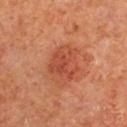The lesion was photographed on a routine skin check and not biopsied; there is no pathology result.
The patient is a male aged 63 to 67.
Imaged with cross-polarized lighting.
Cropped from a total-body skin-imaging series; the visible field is about 15 mm.
Approximately 4 mm at its widest.
The total-body-photography lesion software estimated a mean CIELAB color near L≈46 a*≈31 b*≈34, a lesion–skin lightness drop of about 8, and a normalized border contrast of about 6. The software also gave border irregularity of about 4.5 on a 0–10 scale, a color-variation rating of about 3.5/10, and radial color variation of about 1. The analysis additionally found a nevus-likeness score of about 55/100.
The lesion is on the right upper arm.